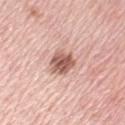size = about 3.5 mm
patient = female, aged around 55
lighting = white-light
imaging modality = ~15 mm crop, total-body skin-cancer survey
body site = the left upper arm